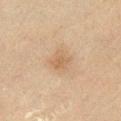Clinical impression:
This lesion was catalogued during total-body skin photography and was not selected for biopsy.
Context:
Located on the left lower leg. The total-body-photography lesion software estimated about 6 CIELAB-L* units darker than the surrounding skin and a normalized lesion–skin contrast near 5.5. The software also gave a border-irregularity index near 3.5/10, a color-variation rating of about 2/10, and radial color variation of about 1. The analysis additionally found an automated nevus-likeness rating near 35 out of 100 and a lesion-detection confidence of about 100/100. A close-up tile cropped from a whole-body skin photograph, about 15 mm across. Approximately 2.5 mm at its widest. A male patient, roughly 65 years of age.